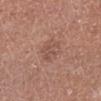Assessment:
The lesion was tiled from a total-body skin photograph and was not biopsied.
Acquisition and patient details:
On the left lower leg. A 15 mm crop from a total-body photograph taken for skin-cancer surveillance. The patient is a male aged 63 to 67.